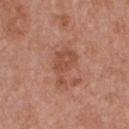The lesion was tiled from a total-body skin photograph and was not biopsied. A female subject in their 40s. Located on the front of the torso. The tile uses white-light illumination. A region of skin cropped from a whole-body photographic capture, roughly 15 mm wide. Longest diameter approximately 5 mm.